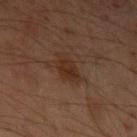No biopsy was performed on this lesion — it was imaged during a full skin examination and was not determined to be concerning. This image is a 15 mm lesion crop taken from a total-body photograph. Automated tile analysis of the lesion measured an average lesion color of about L≈26 a*≈16 b*≈24 (CIELAB) and about 6 CIELAB-L* units darker than the surrounding skin. The analysis additionally found a border-irregularity rating of about 3.5/10, internal color variation of about 2.5 on a 0–10 scale, and peripheral color asymmetry of about 1. It also reported a nevus-likeness score of about 60/100 and a detector confidence of about 100 out of 100 that the crop contains a lesion. Imaged with cross-polarized lighting. A male patient, about 50 years old. The lesion's longest dimension is about 3.5 mm. Located on the left upper arm.